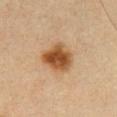The lesion was photographed on a routine skin check and not biopsied; there is no pathology result.
A region of skin cropped from a whole-body photographic capture, roughly 15 mm wide.
The lesion is on the chest.
A male subject, approximately 40 years of age.